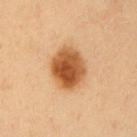Clinical summary: The tile uses cross-polarized illumination. The lesion-visualizer software estimated a mean CIELAB color near L≈55 a*≈25 b*≈42, a lesion–skin lightness drop of about 17, and a lesion-to-skin contrast of about 11.5 (normalized; higher = more distinct). The analysis additionally found a nevus-likeness score of about 100/100 and a lesion-detection confidence of about 100/100. Approximately 5 mm at its widest. A close-up tile cropped from a whole-body skin photograph, about 15 mm across. A male patient aged around 50. The lesion is on the chest.